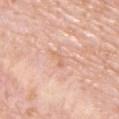On the upper back. About 2.5 mm across. The tile uses white-light illumination. A roughly 15 mm field-of-view crop from a total-body skin photograph. The patient is a female approximately 45 years of age. The lesion-visualizer software estimated an area of roughly 2 mm², an outline eccentricity of about 0.95 (0 = round, 1 = elongated), and a symmetry-axis asymmetry near 0.75. It also reported a mean CIELAB color near L≈68 a*≈23 b*≈32, about 7 CIELAB-L* units darker than the surrounding skin, and a lesion-to-skin contrast of about 5 (normalized; higher = more distinct). The analysis additionally found a border-irregularity rating of about 7/10, internal color variation of about 0 on a 0–10 scale, and radial color variation of about 0.